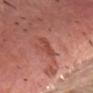Recorded during total-body skin imaging; not selected for excision or biopsy. The lesion is located on the chest. Captured under white-light illumination. A male subject, about 80 years old. A region of skin cropped from a whole-body photographic capture, roughly 15 mm wide. Approximately 3 mm at its widest.